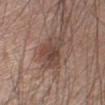The lesion was photographed on a routine skin check and not biopsied; there is no pathology result. This image is a 15 mm lesion crop taken from a total-body photograph. Longest diameter approximately 6 mm. A male subject aged approximately 45. From the right forearm. The tile uses white-light illumination.